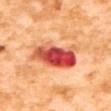Case summary:
– follow-up: total-body-photography surveillance lesion; no biopsy
– acquisition: total-body-photography crop, ~15 mm field of view
– site: the upper back
– subject: female, aged 53–57
– diameter: ~6 mm (longest diameter)
– illumination: cross-polarized